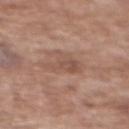Part of a total-body skin-imaging series; this lesion was reviewed on a skin check and was not flagged for biopsy. A 15 mm crop from a total-body photograph taken for skin-cancer surveillance. Longest diameter approximately 4 mm. The subject is a female in their mid- to late 70s. The lesion is on the upper back. Imaged with white-light lighting. An algorithmic analysis of the crop reported an automated nevus-likeness rating near 0 out of 100 and a detector confidence of about 100 out of 100 that the crop contains a lesion.